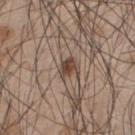Clinical impression: Recorded during total-body skin imaging; not selected for excision or biopsy. Acquisition and patient details: On the upper back. A male patient approximately 45 years of age. A lesion tile, about 15 mm wide, cut from a 3D total-body photograph.